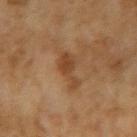Clinical summary: Imaged with cross-polarized lighting. The lesion is located on the left upper arm. Measured at roughly 4 mm in maximum diameter. The patient is a female in their 60s. An algorithmic analysis of the crop reported a lesion area of about 6 mm² and an eccentricity of roughly 0.9. This image is a 15 mm lesion crop taken from a total-body photograph.